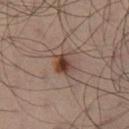Q: Was a biopsy performed?
A: imaged on a skin check; not biopsied
Q: How was this image acquired?
A: ~15 mm crop, total-body skin-cancer survey
Q: What is the lesion's diameter?
A: ≈3 mm
Q: How was the tile lit?
A: cross-polarized
Q: What is the anatomic site?
A: the left leg
Q: Patient demographics?
A: male, about 50 years old